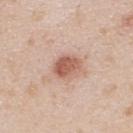  biopsy_status: not biopsied; imaged during a skin examination
  lighting: white-light
  automated_metrics:
    area_mm2_approx: 6.5
    eccentricity: 0.65
    shape_asymmetry: 0.2
    border_irregularity_0_10: 2.0
    color_variation_0_10: 4.0
    peripheral_color_asymmetry: 1.5
  site: upper back
  patient:
    sex: male
    age_approx: 25
  lesion_size:
    long_diameter_mm_approx: 3.5
  image:
    source: total-body photography crop
    field_of_view_mm: 15